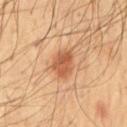Assessment:
Imaged during a routine full-body skin examination; the lesion was not biopsied and no histopathology is available.
Clinical summary:
A 15 mm crop from a total-body photograph taken for skin-cancer surveillance. Captured under cross-polarized illumination. Automated image analysis of the tile measured a footprint of about 7 mm², an eccentricity of roughly 0.7, and two-axis asymmetry of about 0.2. It also reported internal color variation of about 3 on a 0–10 scale. The analysis additionally found a classifier nevus-likeness of about 95/100. Longest diameter approximately 3.5 mm. The subject is a male in their mid-50s. From the chest.